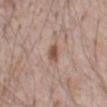Assessment: Captured during whole-body skin photography for melanoma surveillance; the lesion was not biopsied. Image and clinical context: The lesion-visualizer software estimated a lesion color around L≈52 a*≈19 b*≈27 in CIELAB, a lesion–skin lightness drop of about 11, and a lesion-to-skin contrast of about 8.5 (normalized; higher = more distinct). The software also gave a border-irregularity rating of about 2/10, internal color variation of about 2 on a 0–10 scale, and a peripheral color-asymmetry measure near 0.5. The analysis additionally found a nevus-likeness score of about 95/100. The tile uses white-light illumination. Longest diameter approximately 2.5 mm. On the abdomen. Cropped from a total-body skin-imaging series; the visible field is about 15 mm. The patient is a male roughly 70 years of age.